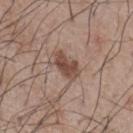Clinical impression:
No biopsy was performed on this lesion — it was imaged during a full skin examination and was not determined to be concerning.
Background:
Approximately 3.5 mm at its widest. A male patient, roughly 75 years of age. Automated tile analysis of the lesion measured a border-irregularity index near 3/10 and internal color variation of about 2.5 on a 0–10 scale. From the chest. A 15 mm crop from a total-body photograph taken for skin-cancer surveillance.